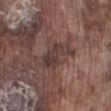| field | value |
|---|---|
| biopsy status | total-body-photography surveillance lesion; no biopsy |
| image-analysis metrics | a lesion color around L≈37 a*≈17 b*≈18 in CIELAB and about 9 CIELAB-L* units darker than the surrounding skin; border irregularity of about 5.5 on a 0–10 scale, a color-variation rating of about 3.5/10, and peripheral color asymmetry of about 1; a classifier nevus-likeness of about 0/100 |
| acquisition | 15 mm crop, total-body photography |
| location | the leg |
| patient | male, aged around 75 |